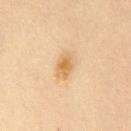Clinical impression: Captured during whole-body skin photography for melanoma surveillance; the lesion was not biopsied. Image and clinical context: Located on the chest. Captured under cross-polarized illumination. An algorithmic analysis of the crop reported an area of roughly 6 mm². The analysis additionally found a normalized border contrast of about 7. The software also gave internal color variation of about 5 on a 0–10 scale and radial color variation of about 1.5. And it measured an automated nevus-likeness rating near 75 out of 100 and lesion-presence confidence of about 100/100. The subject is a male aged approximately 35. A region of skin cropped from a whole-body photographic capture, roughly 15 mm wide.